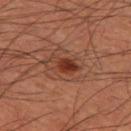Assessment: Imaged during a routine full-body skin examination; the lesion was not biopsied and no histopathology is available. Background: Captured under cross-polarized illumination. A male subject, about 60 years old. Cropped from a total-body skin-imaging series; the visible field is about 15 mm. Located on the left thigh.